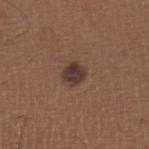Assessment:
The lesion was photographed on a routine skin check and not biopsied; there is no pathology result.
Context:
Cropped from a total-body skin-imaging series; the visible field is about 15 mm. The subject is a male in their mid-60s. From the right lower leg.